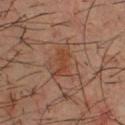Impression: The lesion was tiled from a total-body skin photograph and was not biopsied. Acquisition and patient details: Longest diameter approximately 3 mm. Automated tile analysis of the lesion measured an area of roughly 4 mm², a shape eccentricity near 0.9, and two-axis asymmetry of about 0.4. And it measured a lesion color around L≈38 a*≈22 b*≈29 in CIELAB and about 6 CIELAB-L* units darker than the surrounding skin. It also reported a border-irregularity index near 5/10 and a peripheral color-asymmetry measure near 0. The subject is a male aged approximately 35. The lesion is on the chest. A 15 mm close-up extracted from a 3D total-body photography capture. This is a cross-polarized tile.